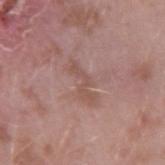This lesion was catalogued during total-body skin photography and was not selected for biopsy. A male subject, aged 38 to 42. This is a white-light tile. Measured at roughly 5 mm in maximum diameter. On the right upper arm. A lesion tile, about 15 mm wide, cut from a 3D total-body photograph.